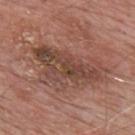This lesion was catalogued during total-body skin photography and was not selected for biopsy.
The tile uses white-light illumination.
The lesion is on the upper back.
A close-up tile cropped from a whole-body skin photograph, about 15 mm across.
The subject is a male aged 78–82.